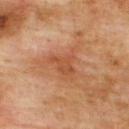{
  "biopsy_status": "not biopsied; imaged during a skin examination",
  "image": {
    "source": "total-body photography crop",
    "field_of_view_mm": 15
  },
  "site": "upper back",
  "patient": {
    "sex": "male",
    "age_approx": 75
  },
  "lesion_size": {
    "long_diameter_mm_approx": 3.0
  }
}